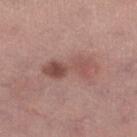* biopsy status: imaged on a skin check; not biopsied
* lighting: white-light
* lesion diameter: ~6 mm (longest diameter)
* subject: female, aged around 55
* imaging modality: ~15 mm crop, total-body skin-cancer survey
* anatomic site: the left lower leg